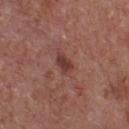notes: imaged on a skin check; not biopsied | body site: the chest | lesion diameter: ≈2.5 mm | patient: male, aged approximately 55 | imaging modality: ~15 mm tile from a whole-body skin photo.